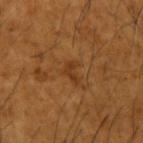This lesion was catalogued during total-body skin photography and was not selected for biopsy. This is a cross-polarized tile. A region of skin cropped from a whole-body photographic capture, roughly 15 mm wide. Longest diameter approximately 2.5 mm. Located on the left forearm. A male subject aged around 65.